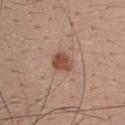site: the head or neck | image source: ~15 mm crop, total-body skin-cancer survey | patient: female, aged approximately 25 | automated metrics: an area of roughly 5 mm² and an outline eccentricity of about 0.65 (0 = round, 1 = elongated); a lesion–skin lightness drop of about 12 and a lesion-to-skin contrast of about 9 (normalized; higher = more distinct); a border-irregularity index near 2/10, internal color variation of about 3 on a 0–10 scale, and peripheral color asymmetry of about 1; lesion-presence confidence of about 100/100 | lighting: white-light illumination | lesion size: ~3 mm (longest diameter).